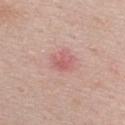| feature | finding |
|---|---|
| notes | total-body-photography surveillance lesion; no biopsy |
| location | the front of the torso |
| imaging modality | 15 mm crop, total-body photography |
| lighting | white-light |
| patient | male, about 60 years old |